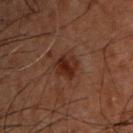follow-up — total-body-photography surveillance lesion; no biopsy | subject — male, aged around 50 | anatomic site — the upper back | imaging modality — ~15 mm tile from a whole-body skin photo.